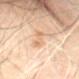  biopsy_status: not biopsied; imaged during a skin examination
  image:
    source: total-body photography crop
    field_of_view_mm: 15
  lighting: cross-polarized
  patient:
    sex: male
    age_approx: 60
  site: lower back
  lesion_size:
    long_diameter_mm_approx: 3.5